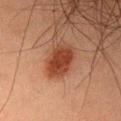Captured during whole-body skin photography for melanoma surveillance; the lesion was not biopsied. An algorithmic analysis of the crop reported a classifier nevus-likeness of about 100/100 and a lesion-detection confidence of about 100/100. This is a cross-polarized tile. A 15 mm crop from a total-body photograph taken for skin-cancer surveillance. About 4.5 mm across. The lesion is on the front of the torso. A male patient in their 50s.